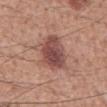Q: Was this lesion biopsied?
A: total-body-photography surveillance lesion; no biopsy
Q: Where on the body is the lesion?
A: the mid back
Q: Illumination type?
A: white-light illumination
Q: What is the lesion's diameter?
A: ~6 mm (longest diameter)
Q: How was this image acquired?
A: ~15 mm crop, total-body skin-cancer survey
Q: Who is the patient?
A: male, aged 53–57